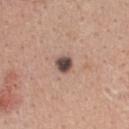Part of a total-body skin-imaging series; this lesion was reviewed on a skin check and was not flagged for biopsy.
The recorded lesion diameter is about 2 mm.
A female patient about 40 years old.
This is a white-light tile.
This image is a 15 mm lesion crop taken from a total-body photograph.
On the upper back.